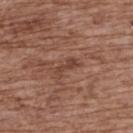biopsy status = total-body-photography surveillance lesion; no biopsy
anatomic site = the upper back
patient = female, in their mid- to late 70s
illumination = white-light
imaging modality = ~15 mm tile from a whole-body skin photo